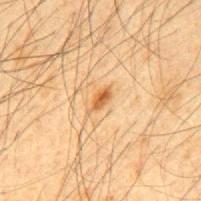No biopsy was performed on this lesion — it was imaged during a full skin examination and was not determined to be concerning. A male subject aged around 65. Located on the mid back. The tile uses cross-polarized illumination. The recorded lesion diameter is about 3 mm. Cropped from a whole-body photographic skin survey; the tile spans about 15 mm.